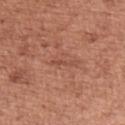biopsy_status: not biopsied; imaged during a skin examination
patient:
  sex: female
  age_approx: 50
lesion_size:
  long_diameter_mm_approx: 3.0
lighting: white-light
site: left upper arm
image:
  source: total-body photography crop
  field_of_view_mm: 15